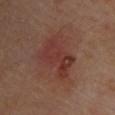Image and clinical context: A male subject, in their mid-60s. From the upper back. Cropped from a total-body skin-imaging series; the visible field is about 15 mm.